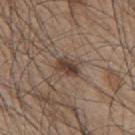Captured during whole-body skin photography for melanoma surveillance; the lesion was not biopsied. A male subject, in their mid- to late 40s. The lesion's longest dimension is about 3 mm. Automated image analysis of the tile measured roughly 11 lightness units darker than nearby skin. The software also gave a border-irregularity index near 2.5/10, a color-variation rating of about 3.5/10, and peripheral color asymmetry of about 1. The analysis additionally found lesion-presence confidence of about 100/100. The tile uses white-light illumination. From the back. A 15 mm crop from a total-body photograph taken for skin-cancer surveillance.